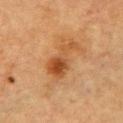Captured during whole-body skin photography for melanoma surveillance; the lesion was not biopsied. The recorded lesion diameter is about 5 mm. A male subject aged approximately 85 or older. On the front of the torso. A lesion tile, about 15 mm wide, cut from a 3D total-body photograph.